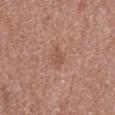<lesion>
<biopsy_status>not biopsied; imaged during a skin examination</biopsy_status>
<patient>
  <sex>female</sex>
  <age_approx>50</age_approx>
</patient>
<image>
  <source>total-body photography crop</source>
  <field_of_view_mm>15</field_of_view_mm>
</image>
<automated_metrics>
  <eccentricity>0.7</eccentricity>
  <shape_asymmetry>0.2</shape_asymmetry>
</automated_metrics>
<lighting>white-light</lighting>
<site>left lower leg</site>
<lesion_size>
  <long_diameter_mm_approx>2.5</long_diameter_mm_approx>
</lesion_size>
</lesion>